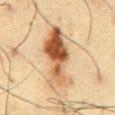Automated tile analysis of the lesion measured a lesion area of about 22 mm² and two-axis asymmetry of about 0.35. It also reported a color-variation rating of about 10/10 and peripheral color asymmetry of about 4.5. It also reported a classifier nevus-likeness of about 95/100.
Imaged with cross-polarized lighting.
A close-up tile cropped from a whole-body skin photograph, about 15 mm across.
About 7.5 mm across.
A male subject, roughly 60 years of age.
The lesion is located on the chest.